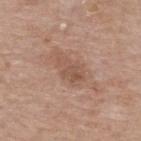TBP lesion metrics: a lesion color around L≈53 a*≈19 b*≈28 in CIELAB, about 8 CIELAB-L* units darker than the surrounding skin, and a normalized lesion–skin contrast near 5.5; border irregularity of about 4 on a 0–10 scale, a within-lesion color-variation index near 3/10, and a peripheral color-asymmetry measure near 1 | subject: male, roughly 85 years of age or older | lighting: white-light | lesion size: about 4 mm | acquisition: 15 mm crop, total-body photography | location: the back.